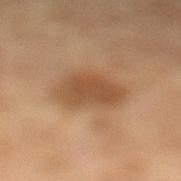biopsy status: no biopsy performed (imaged during a skin exam); tile lighting: cross-polarized; image source: 15 mm crop, total-body photography; site: the left lower leg; lesion size: ~4.5 mm (longest diameter); automated lesion analysis: a lesion area of about 14 mm² and a shape eccentricity near 0.6; patient: female, aged 68–72.